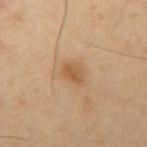<record>
  <biopsy_status>not biopsied; imaged during a skin examination</biopsy_status>
  <image>
    <source>total-body photography crop</source>
    <field_of_view_mm>15</field_of_view_mm>
  </image>
  <patient>
    <sex>male</sex>
    <age_approx>40</age_approx>
  </patient>
  <lesion_size>
    <long_diameter_mm_approx>3.0</long_diameter_mm_approx>
  </lesion_size>
  <site>right upper arm</site>
  <automated_metrics>
    <area_mm2_approx>5.5</area_mm2_approx>
    <eccentricity>0.65</eccentricity>
    <shape_asymmetry>0.25</shape_asymmetry>
    <vs_skin_darker_L>8.0</vs_skin_darker_L>
    <border_irregularity_0_10>2.0</border_irregularity_0_10>
    <color_variation_0_10>4.0</color_variation_0_10>
    <nevus_likeness_0_100>70</nevus_likeness_0_100>
  </automated_metrics>
  <lighting>cross-polarized</lighting>
</record>